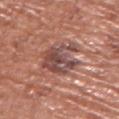TBP lesion metrics: a footprint of about 17 mm², a shape eccentricity near 0.6, and a shape-asymmetry score of about 0.35 (0 = symmetric); a border-irregularity rating of about 5/10, a color-variation rating of about 7/10, and a peripheral color-asymmetry measure near 2.5 | illumination: white-light illumination | location: the chest | imaging modality: 15 mm crop, total-body photography | patient: male, about 75 years old | diameter: about 5.5 mm.